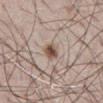Case summary:
* image — ~15 mm tile from a whole-body skin photo
* patient — male, in their 70s
* anatomic site — the front of the torso
* size — about 3 mm
* tile lighting — white-light